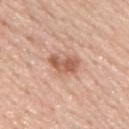{
  "biopsy_status": "not biopsied; imaged during a skin examination",
  "site": "upper back",
  "patient": {
    "sex": "male",
    "age_approx": 45
  },
  "image": {
    "source": "total-body photography crop",
    "field_of_view_mm": 15
  }
}